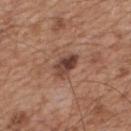follow-up: no biopsy performed (imaged during a skin exam)
size: ≈3.5 mm
patient: male, in their mid-60s
automated lesion analysis: a lesion area of about 6 mm² and two-axis asymmetry of about 0.25; an automated nevus-likeness rating near 50 out of 100 and a detector confidence of about 100 out of 100 that the crop contains a lesion
body site: the mid back
imaging modality: ~15 mm crop, total-body skin-cancer survey